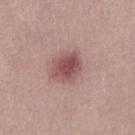{
  "biopsy_status": "not biopsied; imaged during a skin examination",
  "image": {
    "source": "total-body photography crop",
    "field_of_view_mm": 15
  },
  "patient": {
    "sex": "male",
    "age_approx": 30
  }
}